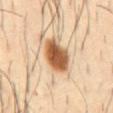follow-up = catalogued during a skin exam; not biopsied
lesion size = ~5 mm (longest diameter)
anatomic site = the abdomen
tile lighting = cross-polarized
subject = male, about 40 years old
image = ~15 mm tile from a whole-body skin photo